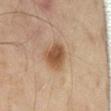Case summary:
- notes · catalogued during a skin exam; not biopsied
- subject · male, approximately 60 years of age
- location · the abdomen
- imaging modality · 15 mm crop, total-body photography